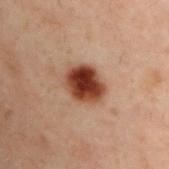Imaged during a routine full-body skin examination; the lesion was not biopsied and no histopathology is available. Automated image analysis of the tile measured a lesion area of about 11 mm², an outline eccentricity of about 0.55 (0 = round, 1 = elongated), and a shape-asymmetry score of about 0.15 (0 = symmetric). The analysis additionally found border irregularity of about 1.5 on a 0–10 scale and peripheral color asymmetry of about 1.5. The analysis additionally found an automated nevus-likeness rating near 100 out of 100 and a lesion-detection confidence of about 100/100. The lesion's longest dimension is about 4 mm. The patient is a male aged around 30. Cropped from a whole-body photographic skin survey; the tile spans about 15 mm. From the upper back.